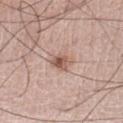Q: Is there a histopathology result?
A: imaged on a skin check; not biopsied
Q: How was this image acquired?
A: ~15 mm crop, total-body skin-cancer survey
Q: What is the lesion's diameter?
A: ~2.5 mm (longest diameter)
Q: What lighting was used for the tile?
A: white-light
Q: What is the anatomic site?
A: the right leg
Q: Patient demographics?
A: male, aged 68 to 72